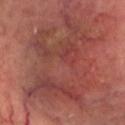patient: male, aged 63–67
anatomic site: the head or neck
imaging modality: ~15 mm tile from a whole-body skin photo
size: ~12.5 mm (longest diameter)
tile lighting: cross-polarized illumination